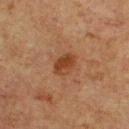Q: Was a biopsy performed?
A: catalogued during a skin exam; not biopsied
Q: What did automated image analysis measure?
A: a footprint of about 5 mm², a shape eccentricity near 0.7, and two-axis asymmetry of about 0.2; a border-irregularity index near 2/10, internal color variation of about 3.5 on a 0–10 scale, and peripheral color asymmetry of about 1.5; a nevus-likeness score of about 85/100 and a detector confidence of about 100 out of 100 that the crop contains a lesion
Q: Illumination type?
A: cross-polarized illumination
Q: What is the anatomic site?
A: the chest
Q: How large is the lesion?
A: about 3 mm
Q: Who is the patient?
A: male, in their mid-70s
Q: What is the imaging modality?
A: ~15 mm tile from a whole-body skin photo